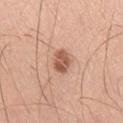No biopsy was performed on this lesion — it was imaged during a full skin examination and was not determined to be concerning.
The lesion-visualizer software estimated an area of roughly 5.5 mm², an outline eccentricity of about 0.75 (0 = round, 1 = elongated), and a symmetry-axis asymmetry near 0.25. The software also gave a border-irregularity rating of about 2.5/10 and a peripheral color-asymmetry measure near 1.5.
A male patient aged 38–42.
Captured under white-light illumination.
A close-up tile cropped from a whole-body skin photograph, about 15 mm across.
About 3 mm across.
On the right upper arm.